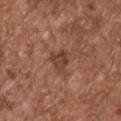| field | value |
|---|---|
| workup | no biopsy performed (imaged during a skin exam) |
| lighting | white-light |
| anatomic site | the chest |
| diameter | about 3 mm |
| imaging modality | ~15 mm tile from a whole-body skin photo |
| subject | male, aged 43–47 |